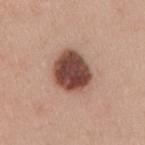notes: no biopsy performed (imaged during a skin exam); imaging modality: ~15 mm crop, total-body skin-cancer survey; patient: male, roughly 35 years of age; body site: the right upper arm.